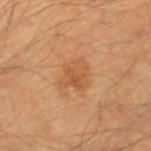Clinical impression: The lesion was tiled from a total-body skin photograph and was not biopsied. Image and clinical context: The lesion-visualizer software estimated border irregularity of about 3 on a 0–10 scale, a within-lesion color-variation index near 3/10, and a peripheral color-asymmetry measure near 1. The patient is a male aged 58 to 62. Longest diameter approximately 3.5 mm. A 15 mm close-up tile from a total-body photography series done for melanoma screening. Imaged with cross-polarized lighting. From the right lower leg.